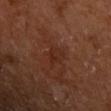Impression: The lesion was photographed on a routine skin check and not biopsied; there is no pathology result. Background: A region of skin cropped from a whole-body photographic capture, roughly 15 mm wide. Approximately 3 mm at its widest. From the left upper arm. Captured under cross-polarized illumination. The subject is a male about 60 years old.